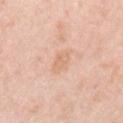Q: Was this lesion biopsied?
A: no biopsy performed (imaged during a skin exam)
Q: What kind of image is this?
A: total-body-photography crop, ~15 mm field of view
Q: What is the anatomic site?
A: the left upper arm
Q: What lighting was used for the tile?
A: white-light
Q: Automated lesion metrics?
A: a shape eccentricity near 0.7 and a symmetry-axis asymmetry near 0.2; roughly 6 lightness units darker than nearby skin and a normalized border contrast of about 5; a nevus-likeness score of about 0/100
Q: What is the lesion's diameter?
A: ≈3 mm
Q: What are the patient's age and sex?
A: female, aged 63–67